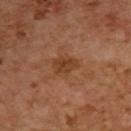Measured at roughly 3 mm in maximum diameter.
The tile uses cross-polarized illumination.
A female patient, about 55 years old.
Located on the upper back.
An algorithmic analysis of the crop reported a mean CIELAB color near L≈41 a*≈23 b*≈34, a lesion–skin lightness drop of about 8, and a lesion-to-skin contrast of about 7 (normalized; higher = more distinct).
A region of skin cropped from a whole-body photographic capture, roughly 15 mm wide.